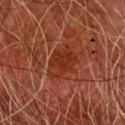No biopsy was performed on this lesion — it was imaged during a full skin examination and was not determined to be concerning. A male patient, aged around 80. Imaged with cross-polarized lighting. Automated image analysis of the tile measured a mean CIELAB color near L≈25 a*≈24 b*≈27, about 6 CIELAB-L* units darker than the surrounding skin, and a lesion-to-skin contrast of about 7.5 (normalized; higher = more distinct). The software also gave a border-irregularity rating of about 2/10, a within-lesion color-variation index near 2.5/10, and radial color variation of about 1. The lesion is on the right forearm. The lesion's longest dimension is about 4 mm. A close-up tile cropped from a whole-body skin photograph, about 15 mm across.